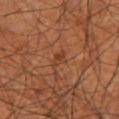Clinical impression:
No biopsy was performed on this lesion — it was imaged during a full skin examination and was not determined to be concerning.
Background:
This image is a 15 mm lesion crop taken from a total-body photograph. From the leg. The patient is a male roughly 70 years of age.